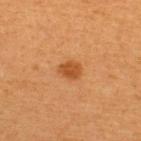notes: total-body-photography surveillance lesion; no biopsy.